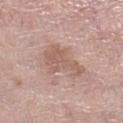Findings:
• follow-up: total-body-photography surveillance lesion; no biopsy
• imaging modality: ~15 mm tile from a whole-body skin photo
• automated lesion analysis: a lesion area of about 11 mm², a shape eccentricity near 0.75, and a shape-asymmetry score of about 0.45 (0 = symmetric); a border-irregularity rating of about 6.5/10, a within-lesion color-variation index near 2/10, and a peripheral color-asymmetry measure near 1; a nevus-likeness score of about 0/100
• lighting: white-light illumination
• body site: the left lower leg
• subject: female, aged 73 to 77